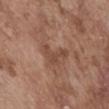– workup — catalogued during a skin exam; not biopsied
– image source — ~15 mm crop, total-body skin-cancer survey
– patient — male, about 75 years old
– location — the abdomen